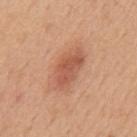Notes:
• follow-up · no biopsy performed (imaged during a skin exam)
• acquisition · ~15 mm tile from a whole-body skin photo
• patient · male, about 50 years old
• location · the mid back
• automated lesion analysis · a mean CIELAB color near L≈56 a*≈26 b*≈33, about 11 CIELAB-L* units darker than the surrounding skin, and a lesion-to-skin contrast of about 7 (normalized; higher = more distinct)